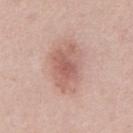Assessment: The lesion was tiled from a total-body skin photograph and was not biopsied. Clinical summary: On the chest. Imaged with white-light lighting. The lesion's longest dimension is about 6 mm. This image is a 15 mm lesion crop taken from a total-body photograph. A male subject in their mid- to late 50s. An algorithmic analysis of the crop reported an average lesion color of about L≈60 a*≈21 b*≈25 (CIELAB), roughly 10 lightness units darker than nearby skin, and a normalized border contrast of about 6.5. And it measured a border-irregularity rating of about 2.5/10, internal color variation of about 5 on a 0–10 scale, and peripheral color asymmetry of about 1.5. And it measured a lesion-detection confidence of about 100/100.